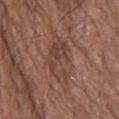Notes:
• image · ~15 mm tile from a whole-body skin photo
• body site · the upper back
• subject · male, about 75 years old